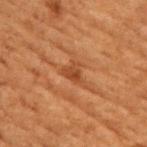notes = catalogued during a skin exam; not biopsied | tile lighting = cross-polarized illumination | lesion diameter = ~2.5 mm (longest diameter) | anatomic site = the chest | acquisition = ~15 mm crop, total-body skin-cancer survey | subject = female, aged around 80.